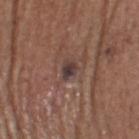Impression:
No biopsy was performed on this lesion — it was imaged during a full skin examination and was not determined to be concerning.
Context:
Automated image analysis of the tile measured a lesion–skin lightness drop of about 10 and a normalized lesion–skin contrast near 9.5. Longest diameter approximately 2.5 mm. Imaged with white-light lighting. A male patient approximately 65 years of age. Cropped from a whole-body photographic skin survey; the tile spans about 15 mm. From the head or neck.